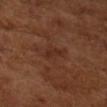{"biopsy_status": "not biopsied; imaged during a skin examination", "patient": {"sex": "male", "age_approx": 65}, "image": {"source": "total-body photography crop", "field_of_view_mm": 15}}